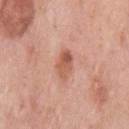Clinical summary: The subject is a female aged 63–67. The lesion is located on the left upper arm. The lesion's longest dimension is about 3 mm. Cropped from a whole-body photographic skin survey; the tile spans about 15 mm.